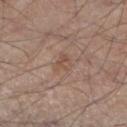follow-up: total-body-photography surveillance lesion; no biopsy
subject: male, aged approximately 45
imaging modality: 15 mm crop, total-body photography
diameter: about 2.5 mm
anatomic site: the leg